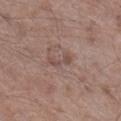Captured during whole-body skin photography for melanoma surveillance; the lesion was not biopsied. A region of skin cropped from a whole-body photographic capture, roughly 15 mm wide. From the leg. The subject is a male aged around 65. The total-body-photography lesion software estimated border irregularity of about 5.5 on a 0–10 scale, a within-lesion color-variation index near 0/10, and radial color variation of about 0. It also reported a nevus-likeness score of about 0/100 and a lesion-detection confidence of about 100/100. About 3 mm across.